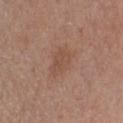follow-up=no biopsy performed (imaged during a skin exam)
site=the chest
subject=male, in their 40s
diameter=~4 mm (longest diameter)
image=~15 mm crop, total-body skin-cancer survey